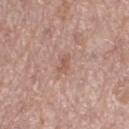Captured during whole-body skin photography for melanoma surveillance; the lesion was not biopsied.
A male subject aged 73 to 77.
Captured under white-light illumination.
Automated tile analysis of the lesion measured a footprint of about 2.5 mm², an outline eccentricity of about 0.9 (0 = round, 1 = elongated), and two-axis asymmetry of about 0.4. It also reported an automated nevus-likeness rating near 0 out of 100 and a detector confidence of about 100 out of 100 that the crop contains a lesion.
Longest diameter approximately 2.5 mm.
The lesion is on the right thigh.
A region of skin cropped from a whole-body photographic capture, roughly 15 mm wide.